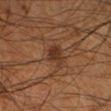Clinical impression:
Imaged during a routine full-body skin examination; the lesion was not biopsied and no histopathology is available.
Clinical summary:
A male subject in their mid-50s. This image is a 15 mm lesion crop taken from a total-body photograph. On the right lower leg. Captured under cross-polarized illumination. About 3 mm across.